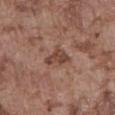The lesion was tiled from a total-body skin photograph and was not biopsied. Cropped from a total-body skin-imaging series; the visible field is about 15 mm. The lesion is located on the front of the torso. Automated image analysis of the tile measured an area of roughly 5.5 mm², an eccentricity of roughly 0.7, and a shape-asymmetry score of about 0.55 (0 = symmetric). And it measured a lesion color around L≈44 a*≈20 b*≈26 in CIELAB, a lesion–skin lightness drop of about 9, and a lesion-to-skin contrast of about 7.5 (normalized; higher = more distinct). And it measured a border-irregularity rating of about 5/10 and peripheral color asymmetry of about 0.5. It also reported a nevus-likeness score of about 0/100. Captured under white-light illumination. The patient is a male aged around 75.